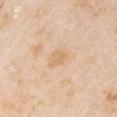workup = imaged on a skin check; not biopsied
image source = ~15 mm crop, total-body skin-cancer survey
lighting = white-light illumination
subject = male, in their mid-70s
anatomic site = the right upper arm